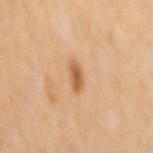{
  "image": {
    "source": "total-body photography crop",
    "field_of_view_mm": 15
  },
  "patient": {
    "sex": "female",
    "age_approx": 55
  },
  "lesion_size": {
    "long_diameter_mm_approx": 3.0
  },
  "site": "back"
}